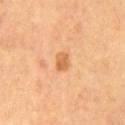workup: imaged on a skin check; not biopsied
lesion size: ~2.5 mm (longest diameter)
automated lesion analysis: roughly 9 lightness units darker than nearby skin; a border-irregularity rating of about 1.5/10, a within-lesion color-variation index near 2/10, and a peripheral color-asymmetry measure near 0.5
anatomic site: the mid back
illumination: cross-polarized illumination
image source: ~15 mm tile from a whole-body skin photo
patient: male, in their mid-60s